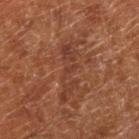Q: Is there a histopathology result?
A: no biopsy performed (imaged during a skin exam)
Q: Automated lesion metrics?
A: a lesion area of about 10 mm², an outline eccentricity of about 0.95 (0 = round, 1 = elongated), and a shape-asymmetry score of about 0.45 (0 = symmetric); a peripheral color-asymmetry measure near 1; a nevus-likeness score of about 0/100 and lesion-presence confidence of about 70/100
Q: Where on the body is the lesion?
A: the right lower leg
Q: How was this image acquired?
A: ~15 mm tile from a whole-body skin photo
Q: What are the patient's age and sex?
A: in their mid- to late 60s